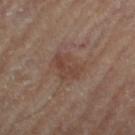Impression: The lesion was tiled from a total-body skin photograph and was not biopsied. Image and clinical context: Automated image analysis of the tile measured a footprint of about 9 mm², a shape eccentricity near 0.6, and two-axis asymmetry of about 0.25. It also reported a mean CIELAB color near L≈40 a*≈17 b*≈24 and roughly 6 lightness units darker than nearby skin. The software also gave internal color variation of about 3 on a 0–10 scale and radial color variation of about 1. The software also gave an automated nevus-likeness rating near 0 out of 100 and lesion-presence confidence of about 100/100. Measured at roughly 3.5 mm in maximum diameter. Captured under cross-polarized illumination. From the left thigh. A male subject aged 83 or older. Cropped from a total-body skin-imaging series; the visible field is about 15 mm.